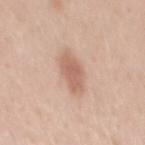Impression: Imaged during a routine full-body skin examination; the lesion was not biopsied and no histopathology is available. Context: Approximately 4.5 mm at its widest. Cropped from a total-body skin-imaging series; the visible field is about 15 mm. Located on the back. The patient is a male aged 33–37.